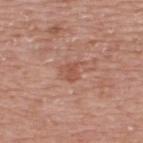Impression: No biopsy was performed on this lesion — it was imaged during a full skin examination and was not determined to be concerning. Image and clinical context: The tile uses white-light illumination. Approximately 3 mm at its widest. Automated tile analysis of the lesion measured an area of roughly 4 mm², an eccentricity of roughly 0.5, and two-axis asymmetry of about 0.4. And it measured a mean CIELAB color near L≈53 a*≈24 b*≈30 and about 7 CIELAB-L* units darker than the surrounding skin. The software also gave a border-irregularity rating of about 4/10, a color-variation rating of about 2/10, and peripheral color asymmetry of about 0.5. And it measured a detector confidence of about 100 out of 100 that the crop contains a lesion. The subject is a male aged 73–77. This image is a 15 mm lesion crop taken from a total-body photograph. The lesion is located on the upper back.